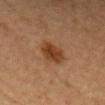notes — no biopsy performed (imaged during a skin exam) | lighting — cross-polarized illumination | imaging modality — ~15 mm crop, total-body skin-cancer survey | size — ~4 mm (longest diameter) | patient — female, aged 43 to 47 | site — the head or neck.